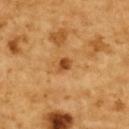A 15 mm close-up tile from a total-body photography series done for melanoma screening.
Approximately 2 mm at its widest.
Located on the upper back.
The subject is a female about 55 years old.
Imaged with cross-polarized lighting.